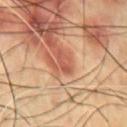Q: Illumination type?
A: cross-polarized illumination
Q: Automated lesion metrics?
A: an average lesion color of about L≈52 a*≈28 b*≈32 (CIELAB), about 10 CIELAB-L* units darker than the surrounding skin, and a lesion-to-skin contrast of about 7 (normalized; higher = more distinct); a border-irregularity rating of about 6/10, a within-lesion color-variation index near 0/10, and a peripheral color-asymmetry measure near 0
Q: How was this image acquired?
A: ~15 mm tile from a whole-body skin photo
Q: What is the lesion's diameter?
A: ≈3 mm
Q: What is the anatomic site?
A: the front of the torso
Q: Who is the patient?
A: male, approximately 70 years of age